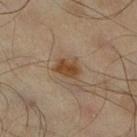Impression: Captured during whole-body skin photography for melanoma surveillance; the lesion was not biopsied. Acquisition and patient details: A roughly 15 mm field-of-view crop from a total-body skin photograph. The recorded lesion diameter is about 3 mm. Automated tile analysis of the lesion measured an average lesion color of about L≈42 a*≈16 b*≈30 (CIELAB) and a lesion–skin lightness drop of about 9. The patient is a male roughly 65 years of age. The lesion is on the right lower leg.